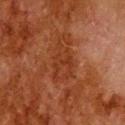Captured during whole-body skin photography for melanoma surveillance; the lesion was not biopsied. About 5.5 mm across. A male subject aged 78–82. Automated image analysis of the tile measured a lesion area of about 11 mm², a shape eccentricity near 0.85, and two-axis asymmetry of about 0.4. The software also gave border irregularity of about 4.5 on a 0–10 scale and a color-variation rating of about 3/10. This is a cross-polarized tile. A roughly 15 mm field-of-view crop from a total-body skin photograph. From the head or neck.